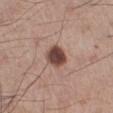Imaged during a routine full-body skin examination; the lesion was not biopsied and no histopathology is available. A lesion tile, about 15 mm wide, cut from a 3D total-body photograph. The total-body-photography lesion software estimated an area of roughly 6.5 mm², an eccentricity of roughly 0.5, and a symmetry-axis asymmetry near 0.15. The analysis additionally found a lesion color around L≈44 a*≈20 b*≈24 in CIELAB and a lesion–skin lightness drop of about 17. A male subject aged approximately 60. Measured at roughly 3 mm in maximum diameter. From the right lower leg.